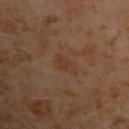Automated image analysis of the tile measured a mean CIELAB color near L≈33 a*≈17 b*≈28, about 4 CIELAB-L* units darker than the surrounding skin, and a lesion-to-skin contrast of about 5.5 (normalized; higher = more distinct). The software also gave an automated nevus-likeness rating near 0 out of 100 and a lesion-detection confidence of about 100/100.
Measured at roughly 3 mm in maximum diameter.
Cropped from a whole-body photographic skin survey; the tile spans about 15 mm.
The patient is a male aged around 60.
The tile uses cross-polarized illumination.
On the upper back.